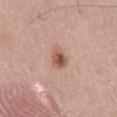{"image": {"source": "total-body photography crop", "field_of_view_mm": 15}, "site": "abdomen", "patient": {"sex": "male", "age_approx": 55}}